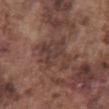Imaged during a routine full-body skin examination; the lesion was not biopsied and no histopathology is available.
A male patient roughly 75 years of age.
The total-body-photography lesion software estimated a lesion color around L≈39 a*≈18 b*≈22 in CIELAB and a normalized border contrast of about 6.5. The software also gave a border-irregularity rating of about 5/10, a within-lesion color-variation index near 3.5/10, and peripheral color asymmetry of about 1.
A roughly 15 mm field-of-view crop from a total-body skin photograph.
Measured at roughly 5 mm in maximum diameter.
This is a white-light tile.
The lesion is located on the abdomen.